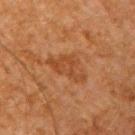Captured during whole-body skin photography for melanoma surveillance; the lesion was not biopsied.
The tile uses cross-polarized illumination.
Measured at roughly 5 mm in maximum diameter.
The lesion is located on the arm.
Cropped from a total-body skin-imaging series; the visible field is about 15 mm.
A male subject, aged approximately 65.